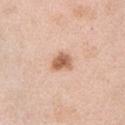Assessment:
Imaged during a routine full-body skin examination; the lesion was not biopsied and no histopathology is available.
Acquisition and patient details:
The tile uses white-light illumination. The lesion is located on the arm. Automated tile analysis of the lesion measured a lesion area of about 4.5 mm², a shape eccentricity near 0.6, and a shape-asymmetry score of about 0.25 (0 = symmetric). The analysis additionally found about 15 CIELAB-L* units darker than the surrounding skin and a normalized lesion–skin contrast near 9. It also reported a border-irregularity index near 2/10 and a color-variation rating of about 3/10. Cropped from a whole-body photographic skin survey; the tile spans about 15 mm. A male patient, in their 60s.